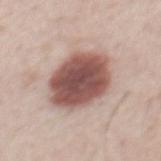follow-up: catalogued during a skin exam; not biopsied
subject: male, in their 40s
illumination: white-light illumination
image source: ~15 mm tile from a whole-body skin photo
automated lesion analysis: a lesion color around L≈52 a*≈21 b*≈23 in CIELAB, roughly 19 lightness units darker than nearby skin, and a lesion-to-skin contrast of about 12.5 (normalized; higher = more distinct); a border-irregularity rating of about 1.5/10 and internal color variation of about 5 on a 0–10 scale
body site: the back